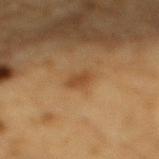Impression: Recorded during total-body skin imaging; not selected for excision or biopsy. Clinical summary: The recorded lesion diameter is about 3 mm. From the mid back. Imaged with cross-polarized lighting. Cropped from a total-body skin-imaging series; the visible field is about 15 mm. An algorithmic analysis of the crop reported a nevus-likeness score of about 10/100 and a lesion-detection confidence of about 100/100. A male subject roughly 85 years of age or older.